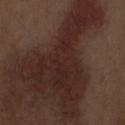notes: total-body-photography surveillance lesion; no biopsy
tile lighting: white-light illumination
anatomic site: the abdomen
diameter: ~14 mm (longest diameter)
acquisition: 15 mm crop, total-body photography
image-analysis metrics: two-axis asymmetry of about 0.75; a border-irregularity index near 10/10; an automated nevus-likeness rating near 0 out of 100 and a detector confidence of about 70 out of 100 that the crop contains a lesion
subject: male, about 70 years old